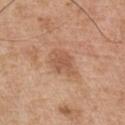notes: total-body-photography surveillance lesion; no biopsy
imaging modality: 15 mm crop, total-body photography
TBP lesion metrics: a lesion area of about 8.5 mm², an outline eccentricity of about 0.75 (0 = round, 1 = elongated), and a symmetry-axis asymmetry near 0.2; a border-irregularity rating of about 2.5/10 and radial color variation of about 0.5; a classifier nevus-likeness of about 0/100 and a lesion-detection confidence of about 100/100
subject: male, approximately 55 years of age
tile lighting: white-light illumination
site: the chest
lesion size: ~4 mm (longest diameter)